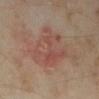Background:
The total-body-photography lesion software estimated a shape eccentricity near 0.6 and two-axis asymmetry of about 0.3. It also reported an average lesion color of about L≈46 a*≈20 b*≈25 (CIELAB), about 6 CIELAB-L* units darker than the surrounding skin, and a normalized border contrast of about 5. It also reported a border-irregularity rating of about 4/10, a color-variation rating of about 5/10, and a peripheral color-asymmetry measure near 1.5. The subject is a female aged 33–37. A roughly 15 mm field-of-view crop from a total-body skin photograph. The lesion is located on the right lower leg. Approximately 6.5 mm at its widest.